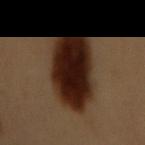Impression:
Imaged during a routine full-body skin examination; the lesion was not biopsied and no histopathology is available.
Clinical summary:
Measured at roughly 9 mm in maximum diameter. A region of skin cropped from a whole-body photographic capture, roughly 15 mm wide. The subject is a female in their 60s. The lesion is located on the mid back. The tile uses cross-polarized illumination.